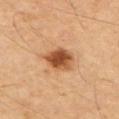follow-up: total-body-photography surveillance lesion; no biopsy.